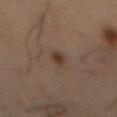Clinical impression: Captured during whole-body skin photography for melanoma surveillance; the lesion was not biopsied. Image and clinical context: The lesion is located on the abdomen. Approximately 2.5 mm at its widest. Cropped from a whole-body photographic skin survey; the tile spans about 15 mm. The tile uses cross-polarized illumination. A male subject, approximately 55 years of age.